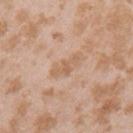Imaged during a routine full-body skin examination; the lesion was not biopsied and no histopathology is available. A female subject aged 23–27. A 15 mm crop from a total-body photograph taken for skin-cancer surveillance. From the left upper arm. Imaged with white-light lighting. Approximately 4 mm at its widest.